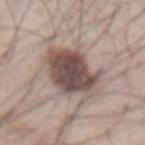This lesion was catalogued during total-body skin photography and was not selected for biopsy. A 15 mm close-up extracted from a 3D total-body photography capture. Located on the abdomen. A male patient, aged 63 to 67.